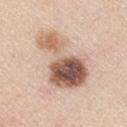Image and clinical context:
A 15 mm crop from a total-body photograph taken for skin-cancer surveillance. The lesion is located on the left upper arm. The subject is a male aged 43–47. Longest diameter approximately 7.5 mm. Automated tile analysis of the lesion measured a footprint of about 24 mm².